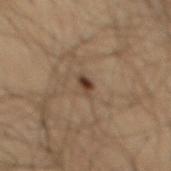biopsy_status: not biopsied; imaged during a skin examination
lesion_size:
  long_diameter_mm_approx: 2.0
patient:
  sex: male
  age_approx: 65
automated_metrics:
  cielab_L: 40
  cielab_a: 16
  cielab_b: 27
  vs_skin_darker_L: 11.0
  nevus_likeness_0_100: 80
  lesion_detection_confidence_0_100: 100
image:
  source: total-body photography crop
  field_of_view_mm: 15
site: mid back